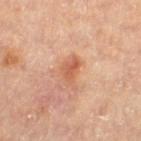Impression: Captured during whole-body skin photography for melanoma surveillance; the lesion was not biopsied. Acquisition and patient details: The lesion-visualizer software estimated a border-irregularity rating of about 3/10, internal color variation of about 4 on a 0–10 scale, and radial color variation of about 1.5. The software also gave an automated nevus-likeness rating near 60 out of 100 and a lesion-detection confidence of about 100/100. Measured at roughly 3 mm in maximum diameter. Captured under cross-polarized illumination. Located on the left thigh. A male subject, aged approximately 85 or older. A close-up tile cropped from a whole-body skin photograph, about 15 mm across.